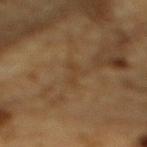Clinical impression: Part of a total-body skin-imaging series; this lesion was reviewed on a skin check and was not flagged for biopsy. Context: On the upper back. The subject is a male in their mid-80s or older. A 15 mm crop from a total-body photograph taken for skin-cancer surveillance.